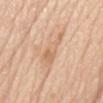Captured during whole-body skin photography for melanoma surveillance; the lesion was not biopsied. A close-up tile cropped from a whole-body skin photograph, about 15 mm across. The lesion-visualizer software estimated a shape-asymmetry score of about 0.45 (0 = symmetric). And it measured a lesion color around L≈67 a*≈19 b*≈35 in CIELAB, about 8 CIELAB-L* units darker than the surrounding skin, and a normalized border contrast of about 5.5. The software also gave a border-irregularity index near 5.5/10. It also reported a nevus-likeness score of about 0/100 and a lesion-detection confidence of about 100/100. Captured under white-light illumination. The lesion is on the abdomen. A male patient aged 73 to 77. Approximately 5.5 mm at its widest.